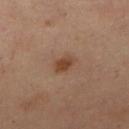Imaged during a routine full-body skin examination; the lesion was not biopsied and no histopathology is available. Captured under cross-polarized illumination. The patient is a female in their mid- to late 50s. From the front of the torso. A region of skin cropped from a whole-body photographic capture, roughly 15 mm wide. Approximately 2.5 mm at its widest.